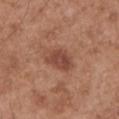Image and clinical context:
The lesion's longest dimension is about 4 mm. The subject is a male aged around 55. The lesion is located on the left upper arm. Cropped from a total-body skin-imaging series; the visible field is about 15 mm.